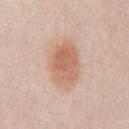Q: Was a biopsy performed?
A: no biopsy performed (imaged during a skin exam)
Q: Illumination type?
A: white-light illumination
Q: Patient demographics?
A: male, aged approximately 25
Q: What is the imaging modality?
A: ~15 mm tile from a whole-body skin photo
Q: Where on the body is the lesion?
A: the chest